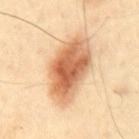<lesion>
  <biopsy_status>not biopsied; imaged during a skin examination</biopsy_status>
  <site>back</site>
  <patient>
    <sex>male</sex>
    <age_approx>65</age_approx>
  </patient>
  <lesion_size>
    <long_diameter_mm_approx>8.0</long_diameter_mm_approx>
  </lesion_size>
  <automated_metrics>
    <vs_skin_darker_L>17.0</vs_skin_darker_L>
    <vs_skin_contrast_norm>10.0</vs_skin_contrast_norm>
  </automated_metrics>
  <image>
    <source>total-body photography crop</source>
    <field_of_view_mm>15</field_of_view_mm>
  </image>
</lesion>